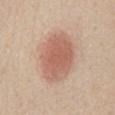Captured during whole-body skin photography for melanoma surveillance; the lesion was not biopsied. About 6.5 mm across. The total-body-photography lesion software estimated an area of roughly 22 mm², a shape eccentricity near 0.75, and a symmetry-axis asymmetry near 0.1. A male subject aged 58–62. The lesion is on the abdomen. A region of skin cropped from a whole-body photographic capture, roughly 15 mm wide.